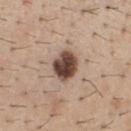Imaged during a routine full-body skin examination; the lesion was not biopsied and no histopathology is available. The tile uses white-light illumination. A 15 mm close-up extracted from a 3D total-body photography capture. On the chest. The patient is a male approximately 40 years of age. Longest diameter approximately 3.5 mm.